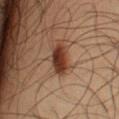Case summary:
* biopsy status: total-body-photography surveillance lesion; no biopsy
* patient: male, aged 48–52
* image: ~15 mm tile from a whole-body skin photo
* body site: the left forearm
* illumination: cross-polarized